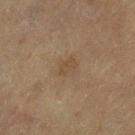Q: What are the patient's age and sex?
A: female, roughly 80 years of age
Q: How large is the lesion?
A: about 2.5 mm
Q: Illumination type?
A: cross-polarized illumination
Q: How was this image acquired?
A: ~15 mm tile from a whole-body skin photo
Q: Where on the body is the lesion?
A: the left thigh